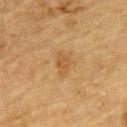Notes:
- workup: catalogued during a skin exam; not biopsied
- imaging modality: total-body-photography crop, ~15 mm field of view
- automated metrics: an area of roughly 3.5 mm², a shape eccentricity near 0.8, and two-axis asymmetry of about 0.25; a lesion color around L≈47 a*≈17 b*≈38 in CIELAB and a lesion–skin lightness drop of about 7; a border-irregularity index near 2.5/10, a color-variation rating of about 1/10, and radial color variation of about 0.5
- diameter: ≈3 mm
- subject: male, aged around 85 or older
- illumination: cross-polarized
- body site: the upper back